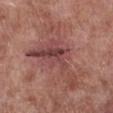image source: 15 mm crop, total-body photography
subject: male, approximately 55 years of age
TBP lesion metrics: a lesion area of about 29 mm², a shape eccentricity near 0.85, and a shape-asymmetry score of about 0.65 (0 = symmetric); a lesion color around L≈46 a*≈24 b*≈23 in CIELAB, about 8 CIELAB-L* units darker than the surrounding skin, and a normalized border contrast of about 6.5; a border-irregularity index near 9.5/10, internal color variation of about 7.5 on a 0–10 scale, and peripheral color asymmetry of about 2.5; an automated nevus-likeness rating near 0 out of 100 and a lesion-detection confidence of about 85/100
lighting: white-light
body site: the right lower leg